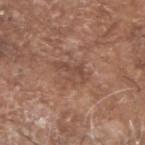notes — no biopsy performed (imaged during a skin exam); patient — male, aged approximately 80; diameter — ≈4 mm; acquisition — ~15 mm tile from a whole-body skin photo; tile lighting — white-light; location — the head or neck.